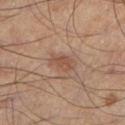Captured during whole-body skin photography for melanoma surveillance; the lesion was not biopsied. A 15 mm crop from a total-body photograph taken for skin-cancer surveillance. An algorithmic analysis of the crop reported a border-irregularity index near 3.5/10 and radial color variation of about 0.5. Captured under cross-polarized illumination. A male subject, about 55 years old. Located on the left lower leg.